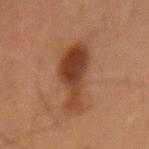Q: Is there a histopathology result?
A: imaged on a skin check; not biopsied
Q: Lesion size?
A: about 7.5 mm
Q: What are the patient's age and sex?
A: male, aged around 65
Q: What kind of image is this?
A: total-body-photography crop, ~15 mm field of view
Q: What did automated image analysis measure?
A: a lesion area of about 16 mm² and a shape-asymmetry score of about 0.45 (0 = symmetric); a lesion color around L≈33 a*≈19 b*≈27 in CIELAB, roughly 11 lightness units darker than nearby skin, and a normalized border contrast of about 10; a border-irregularity index near 6/10, a color-variation rating of about 4.5/10, and a peripheral color-asymmetry measure near 1.5
Q: How was the tile lit?
A: cross-polarized
Q: What is the anatomic site?
A: the back